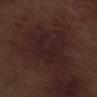Q: Was a biopsy performed?
A: no biopsy performed (imaged during a skin exam)
Q: Lesion location?
A: the right lower leg
Q: What kind of image is this?
A: ~15 mm tile from a whole-body skin photo
Q: What lighting was used for the tile?
A: white-light
Q: What did automated image analysis measure?
A: a footprint of about 19 mm², an eccentricity of roughly 0.25, and a shape-asymmetry score of about 0.35 (0 = symmetric); an average lesion color of about L≈20 a*≈16 b*≈15 (CIELAB) and a lesion–skin lightness drop of about 3; an automated nevus-likeness rating near 0 out of 100 and a detector confidence of about 90 out of 100 that the crop contains a lesion
Q: What is the lesion's diameter?
A: ≈5 mm
Q: What are the patient's age and sex?
A: male, aged approximately 70